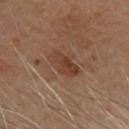Q: Is there a histopathology result?
A: catalogued during a skin exam; not biopsied
Q: What kind of image is this?
A: total-body-photography crop, ~15 mm field of view
Q: Lesion size?
A: about 4.5 mm
Q: Where on the body is the lesion?
A: the head or neck
Q: Who is the patient?
A: female, aged 58 to 62
Q: What lighting was used for the tile?
A: cross-polarized